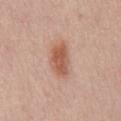notes — no biopsy performed (imaged during a skin exam)
imaging modality — ~15 mm tile from a whole-body skin photo
subject — female, aged 58 to 62
size — ~4 mm (longest diameter)
site — the mid back
lighting — white-light